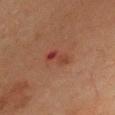From the front of the torso.
Captured under cross-polarized illumination.
This image is a 15 mm lesion crop taken from a total-body photograph.
A male patient, aged 68 to 72.
About 3.5 mm across.